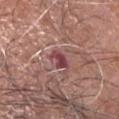Clinical impression:
The lesion was tiled from a total-body skin photograph and was not biopsied.
Image and clinical context:
The patient is a male aged around 65. Measured at roughly 2.5 mm in maximum diameter. A 15 mm close-up extracted from a 3D total-body photography capture. Located on the head or neck. Captured under white-light illumination.